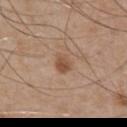Imaged during a routine full-body skin examination; the lesion was not biopsied and no histopathology is available.
About 3 mm across.
From the front of the torso.
Cropped from a whole-body photographic skin survey; the tile spans about 15 mm.
Automated tile analysis of the lesion measured an area of roughly 4 mm² and an eccentricity of roughly 0.75. And it measured a border-irregularity index near 2/10 and internal color variation of about 3.5 on a 0–10 scale. And it measured a classifier nevus-likeness of about 70/100 and a detector confidence of about 100 out of 100 that the crop contains a lesion.
A male patient in their mid- to late 60s.
This is a white-light tile.